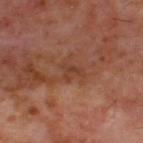Captured under cross-polarized illumination. A male subject aged around 60. The lesion is on the back. The lesion's longest dimension is about 2.5 mm. A roughly 15 mm field-of-view crop from a total-body skin photograph.